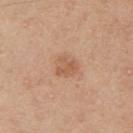<tbp_lesion>
<biopsy_status>not biopsied; imaged during a skin examination</biopsy_status>
<patient>
  <sex>male</sex>
  <age_approx>30</age_approx>
</patient>
<image>
  <source>total-body photography crop</source>
  <field_of_view_mm>15</field_of_view_mm>
</image>
<lighting>white-light</lighting>
<site>right upper arm</site>
<automated_metrics>
  <area_mm2_approx>5.0</area_mm2_approx>
  <shape_asymmetry>0.3</shape_asymmetry>
  <vs_skin_contrast_norm>6.0</vs_skin_contrast_norm>
  <border_irregularity_0_10>3.0</border_irregularity_0_10>
  <color_variation_0_10>2.0</color_variation_0_10>
  <lesion_detection_confidence_0_100>100</lesion_detection_confidence_0_100>
</automated_metrics>
<lesion_size>
  <long_diameter_mm_approx>2.5</long_diameter_mm_approx>
</lesion_size>
</tbp_lesion>